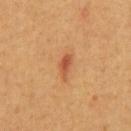Impression: Imaged during a routine full-body skin examination; the lesion was not biopsied and no histopathology is available. Image and clinical context: An algorithmic analysis of the crop reported a lesion area of about 3.5 mm² and two-axis asymmetry of about 0.3. The software also gave a nevus-likeness score of about 70/100 and a detector confidence of about 100 out of 100 that the crop contains a lesion. A male patient aged approximately 60. The lesion's longest dimension is about 3 mm. The lesion is located on the abdomen. This image is a 15 mm lesion crop taken from a total-body photograph.